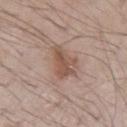biopsy_status: not biopsied; imaged during a skin examination
image:
  source: total-body photography crop
  field_of_view_mm: 15
site: left upper arm
lesion_size:
  long_diameter_mm_approx: 5.5
lighting: white-light
patient:
  sex: male
  age_approx: 70